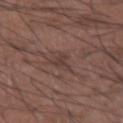Q: Was a biopsy performed?
A: no biopsy performed (imaged during a skin exam)
Q: How large is the lesion?
A: about 3 mm
Q: Patient demographics?
A: male, aged around 50
Q: Lesion location?
A: the right forearm
Q: What did automated image analysis measure?
A: a lesion–skin lightness drop of about 6 and a normalized lesion–skin contrast near 5.5
Q: What kind of image is this?
A: ~15 mm tile from a whole-body skin photo